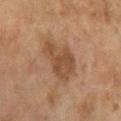notes: total-body-photography surveillance lesion; no biopsy | imaging modality: ~15 mm crop, total-body skin-cancer survey | site: the left forearm | patient: female, aged approximately 60.